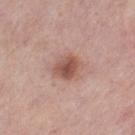Q: Is there a histopathology result?
A: imaged on a skin check; not biopsied
Q: Who is the patient?
A: female, in their 40s
Q: What lighting was used for the tile?
A: white-light
Q: Automated lesion metrics?
A: a lesion area of about 7 mm² and an outline eccentricity of about 0.45 (0 = round, 1 = elongated); border irregularity of about 2 on a 0–10 scale, internal color variation of about 4 on a 0–10 scale, and radial color variation of about 1; a nevus-likeness score of about 85/100 and a lesion-detection confidence of about 100/100
Q: How large is the lesion?
A: about 3 mm
Q: Where on the body is the lesion?
A: the left thigh
Q: How was this image acquired?
A: total-body-photography crop, ~15 mm field of view